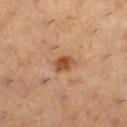Part of a total-body skin-imaging series; this lesion was reviewed on a skin check and was not flagged for biopsy. The tile uses cross-polarized illumination. Measured at roughly 3 mm in maximum diameter. The subject is a female in their 30s. A 15 mm close-up tile from a total-body photography series done for melanoma screening. From the right lower leg.